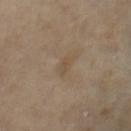Impression:
This lesion was catalogued during total-body skin photography and was not selected for biopsy.
Acquisition and patient details:
About 2.5 mm across. Captured under cross-polarized illumination. The patient is a female in their mid- to late 70s. On the leg. Automated image analysis of the tile measured a mean CIELAB color near L≈49 a*≈13 b*≈29, roughly 5 lightness units darker than nearby skin, and a normalized border contrast of about 5. And it measured a classifier nevus-likeness of about 0/100 and a lesion-detection confidence of about 100/100. Cropped from a whole-body photographic skin survey; the tile spans about 15 mm.